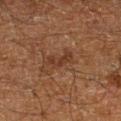biopsy status: no biopsy performed (imaged during a skin exam)
subject: male, aged 58–62
illumination: cross-polarized illumination
site: the right lower leg
lesion size: ≈3.5 mm
acquisition: total-body-photography crop, ~15 mm field of view